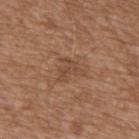{"biopsy_status": "not biopsied; imaged during a skin examination", "patient": {"sex": "male", "age_approx": 75}, "image": {"source": "total-body photography crop", "field_of_view_mm": 15}, "site": "upper back", "automated_metrics": {"area_mm2_approx": 5.0, "eccentricity": 0.5, "border_irregularity_0_10": 7.0, "color_variation_0_10": 2.0, "peripheral_color_asymmetry": 1.0, "nevus_likeness_0_100": 0, "lesion_detection_confidence_0_100": 100}, "lighting": "white-light", "lesion_size": {"long_diameter_mm_approx": 3.5}}